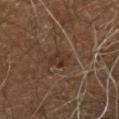Notes:
– workup: catalogued during a skin exam; not biopsied
– body site: the right leg
– automated lesion analysis: a lesion area of about 4.5 mm², a shape eccentricity near 0.7, and a symmetry-axis asymmetry near 0.25; roughly 6 lightness units darker than nearby skin and a normalized lesion–skin contrast near 6; border irregularity of about 2.5 on a 0–10 scale, a within-lesion color-variation index near 2.5/10, and radial color variation of about 1
– imaging modality: ~15 mm tile from a whole-body skin photo
– patient: male, in their 60s
– tile lighting: cross-polarized